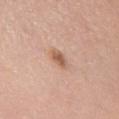Q: Was a biopsy performed?
A: imaged on a skin check; not biopsied
Q: What are the patient's age and sex?
A: female, about 60 years old
Q: How large is the lesion?
A: about 2.5 mm
Q: Lesion location?
A: the chest
Q: How was this image acquired?
A: 15 mm crop, total-body photography